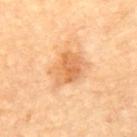The lesion was photographed on a routine skin check and not biopsied; there is no pathology result. A male patient, in their mid-80s or older. About 4.5 mm across. This is a cross-polarized tile. A region of skin cropped from a whole-body photographic capture, roughly 15 mm wide. On the upper back.